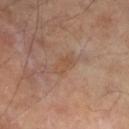Q: Was a biopsy performed?
A: no biopsy performed (imaged during a skin exam)